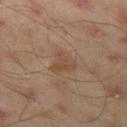Findings:
– biopsy status · total-body-photography surveillance lesion; no biopsy
– lighting · cross-polarized illumination
– image source · 15 mm crop, total-body photography
– diameter · ~3 mm (longest diameter)
– image-analysis metrics · a lesion area of about 5 mm², an eccentricity of roughly 0.4, and two-axis asymmetry of about 0.55; a lesion color around L≈46 a*≈16 b*≈29 in CIELAB and a normalized lesion–skin contrast near 5.5
– anatomic site · the left thigh
– patient · male, approximately 45 years of age